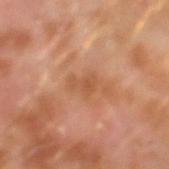The lesion was photographed on a routine skin check and not biopsied; there is no pathology result. Cropped from a total-body skin-imaging series; the visible field is about 15 mm. This is a cross-polarized tile. A male subject aged around 30. From the left forearm. The lesion's longest dimension is about 3 mm. The total-body-photography lesion software estimated a shape eccentricity near 0.8 and two-axis asymmetry of about 0.45. It also reported a border-irregularity rating of about 4.5/10, a color-variation rating of about 1/10, and radial color variation of about 0. It also reported a detector confidence of about 100 out of 100 that the crop contains a lesion.